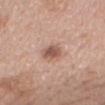– anatomic site — the mid back
– imaging modality — total-body-photography crop, ~15 mm field of view
– patient — female, aged 58 to 62